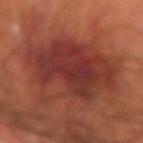Recorded during total-body skin imaging; not selected for excision or biopsy.
Automated tile analysis of the lesion measured a lesion color around L≈35 a*≈27 b*≈26 in CIELAB, a lesion–skin lightness drop of about 10, and a normalized lesion–skin contrast near 9.5. And it measured a nevus-likeness score of about 5/100 and a lesion-detection confidence of about 100/100.
From the left forearm.
A male patient, aged 68 to 72.
This image is a 15 mm lesion crop taken from a total-body photograph.